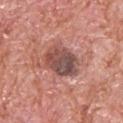follow-up=total-body-photography surveillance lesion; no biopsy
tile lighting=white-light illumination
subject=male, about 80 years old
body site=the head or neck
diameter=≈4.5 mm
image=~15 mm crop, total-body skin-cancer survey